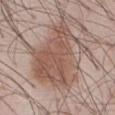Imaged during a routine full-body skin examination; the lesion was not biopsied and no histopathology is available. The total-body-photography lesion software estimated a footprint of about 41 mm², a shape eccentricity near 0.8, and a shape-asymmetry score of about 0.4 (0 = symmetric). The software also gave border irregularity of about 8 on a 0–10 scale and a within-lesion color-variation index near 4/10. Located on the front of the torso. Longest diameter approximately 10.5 mm. A region of skin cropped from a whole-body photographic capture, roughly 15 mm wide. The subject is a male approximately 55 years of age. The tile uses white-light illumination.